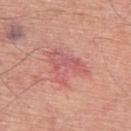notes=no biopsy performed (imaged during a skin exam) | automated lesion analysis=a border-irregularity index near 7/10 and a peripheral color-asymmetry measure near 1.5; a nevus-likeness score of about 0/100 and lesion-presence confidence of about 100/100 | site=the upper back | lesion diameter=~5.5 mm (longest diameter) | subject=male, about 60 years old | tile lighting=white-light illumination | imaging modality=~15 mm crop, total-body skin-cancer survey.